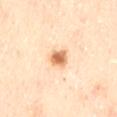The lesion was tiled from a total-body skin photograph and was not biopsied. About 3 mm across. Located on the lower back. A close-up tile cropped from a whole-body skin photograph, about 15 mm across. The tile uses cross-polarized illumination. The subject is a female aged approximately 60.